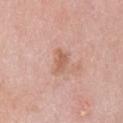Notes:
– follow-up · total-body-photography surveillance lesion; no biopsy
– patient · male, aged around 80
– illumination · white-light illumination
– anatomic site · the mid back
– image · total-body-photography crop, ~15 mm field of view